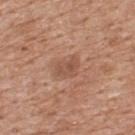Case summary:
• biopsy status · catalogued during a skin exam; not biopsied
• acquisition · total-body-photography crop, ~15 mm field of view
• subject · male, aged approximately 60
• site · the upper back
• size · about 3 mm